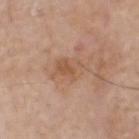  biopsy_status: not biopsied; imaged during a skin examination
  lighting: white-light
  automated_metrics:
    border_irregularity_0_10: 4.0
    color_variation_0_10: 4.5
    peripheral_color_asymmetry: 1.5
    nevus_likeness_0_100: 0
  site: head or neck
  image:
    source: total-body photography crop
    field_of_view_mm: 15
  patient:
    sex: male
    age_approx: 70